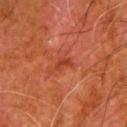Q: Was this lesion biopsied?
A: no biopsy performed (imaged during a skin exam)
Q: What kind of image is this?
A: total-body-photography crop, ~15 mm field of view
Q: Patient demographics?
A: male, aged 78–82
Q: What is the anatomic site?
A: the left upper arm
Q: What did automated image analysis measure?
A: a lesion area of about 2.5 mm² and two-axis asymmetry of about 0.4; a mean CIELAB color near L≈33 a*≈28 b*≈32, roughly 6 lightness units darker than nearby skin, and a lesion-to-skin contrast of about 6.5 (normalized; higher = more distinct); an automated nevus-likeness rating near 0 out of 100 and a lesion-detection confidence of about 100/100
Q: What is the lesion's diameter?
A: ≈2.5 mm